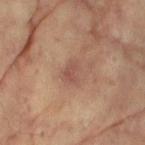follow-up — total-body-photography surveillance lesion; no biopsy | automated metrics — a footprint of about 4.5 mm² and a shape eccentricity near 0.7; an average lesion color of about L≈46 a*≈19 b*≈24 (CIELAB) and a lesion–skin lightness drop of about 7 | image source — ~15 mm crop, total-body skin-cancer survey | illumination — cross-polarized | patient — female, aged 78–82 | body site — the left arm | diameter — ~3 mm (longest diameter).